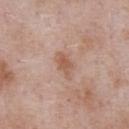image source: ~15 mm crop, total-body skin-cancer survey
TBP lesion metrics: an eccentricity of roughly 0.85 and a shape-asymmetry score of about 0.35 (0 = symmetric); a lesion–skin lightness drop of about 9 and a normalized border contrast of about 6.5; border irregularity of about 3.5 on a 0–10 scale and radial color variation of about 0.5
body site: the chest
illumination: white-light
subject: male, roughly 55 years of age